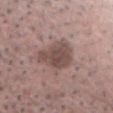This lesion was catalogued during total-body skin photography and was not selected for biopsy. The lesion's longest dimension is about 5 mm. The lesion is on the chest. Captured under white-light illumination. Automated image analysis of the tile measured an outline eccentricity of about 0.7 (0 = round, 1 = elongated). And it measured about 11 CIELAB-L* units darker than the surrounding skin and a normalized lesion–skin contrast near 8. It also reported a border-irregularity index near 3/10 and a color-variation rating of about 3.5/10. The analysis additionally found a classifier nevus-likeness of about 30/100 and a detector confidence of about 100 out of 100 that the crop contains a lesion. Cropped from a whole-body photographic skin survey; the tile spans about 15 mm. The subject is a male approximately 70 years of age.